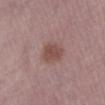Impression:
The lesion was tiled from a total-body skin photograph and was not biopsied.
Clinical summary:
An algorithmic analysis of the crop reported an average lesion color of about L≈48 a*≈20 b*≈22 (CIELAB), a lesion–skin lightness drop of about 9, and a normalized lesion–skin contrast near 7.5. The software also gave border irregularity of about 1.5 on a 0–10 scale, a within-lesion color-variation index near 2/10, and a peripheral color-asymmetry measure near 0.5. And it measured an automated nevus-likeness rating near 65 out of 100 and lesion-presence confidence of about 100/100. The tile uses white-light illumination. A 15 mm crop from a total-body photograph taken for skin-cancer surveillance. Longest diameter approximately 3 mm. Located on the left lower leg. A female subject, roughly 60 years of age.